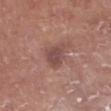Case summary:
* follow-up · no biopsy performed (imaged during a skin exam)
* subject · male, approximately 55 years of age
* image-analysis metrics · a footprint of about 7.5 mm², a shape eccentricity near 0.7, and two-axis asymmetry of about 0.3; an average lesion color of about L≈47 a*≈21 b*≈21 (CIELAB), roughly 9 lightness units darker than nearby skin, and a normalized lesion–skin contrast near 7
* location · the left lower leg
* lesion size · about 4 mm
* imaging modality · total-body-photography crop, ~15 mm field of view